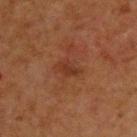No biopsy was performed on this lesion — it was imaged during a full skin examination and was not determined to be concerning. A close-up tile cropped from a whole-body skin photograph, about 15 mm across. Longest diameter approximately 3 mm. Located on the upper back. A male patient aged approximately 65. The lesion-visualizer software estimated a classifier nevus-likeness of about 0/100.